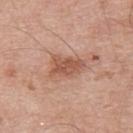Impression:
Part of a total-body skin-imaging series; this lesion was reviewed on a skin check and was not flagged for biopsy.
Image and clinical context:
The patient is a male aged approximately 55. Measured at roughly 4 mm in maximum diameter. Imaged with white-light lighting. A 15 mm crop from a total-body photograph taken for skin-cancer surveillance. The lesion is on the upper back.